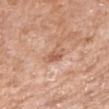This lesion was catalogued during total-body skin photography and was not selected for biopsy.